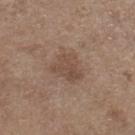  biopsy_status: not biopsied; imaged during a skin examination
  lesion_size:
    long_diameter_mm_approx: 3.5
  image:
    source: total-body photography crop
    field_of_view_mm: 15
  site: right lower leg
  automated_metrics:
    area_mm2_approx: 8.5
    eccentricity: 0.55
    shape_asymmetry: 0.35
    color_variation_0_10: 3.0
    nevus_likeness_0_100: 0
    lesion_detection_confidence_0_100: 100
  patient:
    sex: female
    age_approx: 65